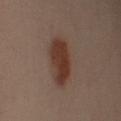Q: Was a biopsy performed?
A: catalogued during a skin exam; not biopsied
Q: What is the anatomic site?
A: the left upper arm
Q: What kind of image is this?
A: total-body-photography crop, ~15 mm field of view
Q: How was the tile lit?
A: cross-polarized
Q: Who is the patient?
A: male, about 50 years old